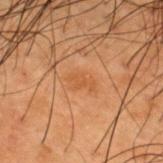Assessment:
Recorded during total-body skin imaging; not selected for excision or biopsy.
Acquisition and patient details:
The lesion is located on the back. A male patient approximately 50 years of age. A roughly 15 mm field-of-view crop from a total-body skin photograph.